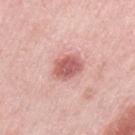Q: Was a biopsy performed?
A: total-body-photography surveillance lesion; no biopsy